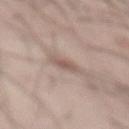Q: Was this lesion biopsied?
A: imaged on a skin check; not biopsied
Q: Who is the patient?
A: male, aged approximately 55
Q: Lesion location?
A: the abdomen
Q: What kind of image is this?
A: total-body-photography crop, ~15 mm field of view
Q: Automated lesion metrics?
A: border irregularity of about 2.5 on a 0–10 scale, internal color variation of about 2 on a 0–10 scale, and radial color variation of about 0.5; a classifier nevus-likeness of about 5/100 and a lesion-detection confidence of about 95/100
Q: What is the lesion's diameter?
A: ~2.5 mm (longest diameter)